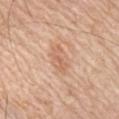follow-up: total-body-photography surveillance lesion; no biopsy
subject: male, about 55 years old
image source: 15 mm crop, total-body photography
lighting: white-light
anatomic site: the front of the torso